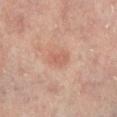Case summary:
– follow-up · catalogued during a skin exam; not biopsied
– body site · the right lower leg
– image source · 15 mm crop, total-body photography
– automated metrics · a border-irregularity rating of about 2/10, a within-lesion color-variation index near 1.5/10, and a peripheral color-asymmetry measure near 0.5
– lighting · cross-polarized illumination
– patient · male, approximately 65 years of age